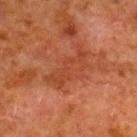A male subject aged around 80.
A 15 mm close-up extracted from a 3D total-body photography capture.
The recorded lesion diameter is about 6 mm.
Imaged with cross-polarized lighting.
The lesion is located on the right lower leg.